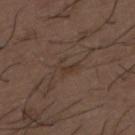| feature | finding |
|---|---|
| notes | no biopsy performed (imaged during a skin exam) |
| diameter | ~2.5 mm (longest diameter) |
| imaging modality | ~15 mm tile from a whole-body skin photo |
| subject | male, aged approximately 50 |
| site | the mid back |